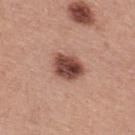– notes · total-body-photography surveillance lesion; no biopsy
– image source · 15 mm crop, total-body photography
– patient · male, about 40 years old
– site · the back